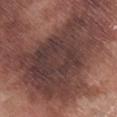Q: Was this lesion biopsied?
A: catalogued during a skin exam; not biopsied
Q: What kind of image is this?
A: ~15 mm crop, total-body skin-cancer survey
Q: Patient demographics?
A: male, about 75 years old
Q: Where on the body is the lesion?
A: the right lower leg